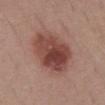Context:
Automated tile analysis of the lesion measured a footprint of about 24 mm² and an eccentricity of roughly 0.65. The software also gave a lesion color around L≈45 a*≈23 b*≈24 in CIELAB and a lesion-to-skin contrast of about 9.5 (normalized; higher = more distinct). It also reported a border-irregularity index near 2/10, internal color variation of about 7 on a 0–10 scale, and a peripheral color-asymmetry measure near 2.5. The software also gave a classifier nevus-likeness of about 75/100 and a detector confidence of about 100 out of 100 that the crop contains a lesion. On the abdomen. A 15 mm close-up extracted from a 3D total-body photography capture. A male patient aged around 40.
Diagnosis:
Histopathology of the biopsied lesion showed an invasive melanoma, superficial spreading type; Breslow depth 0.4 mm — a malignant lesion.